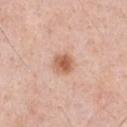Assessment:
Part of a total-body skin-imaging series; this lesion was reviewed on a skin check and was not flagged for biopsy.
Clinical summary:
A 15 mm close-up extracted from a 3D total-body photography capture. A male subject, aged 48–52. About 2.5 mm across. The tile uses white-light illumination. The lesion is on the chest. An algorithmic analysis of the crop reported a footprint of about 5.5 mm², an eccentricity of roughly 0.4, and a shape-asymmetry score of about 0.15 (0 = symmetric). The software also gave a color-variation rating of about 3.5/10 and peripheral color asymmetry of about 1.